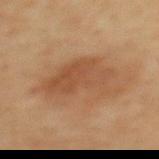Q: Was this lesion biopsied?
A: catalogued during a skin exam; not biopsied
Q: What are the patient's age and sex?
A: female, approximately 55 years of age
Q: Where on the body is the lesion?
A: the back
Q: What is the lesion's diameter?
A: ~8 mm (longest diameter)
Q: How was this image acquired?
A: ~15 mm crop, total-body skin-cancer survey
Q: What lighting was used for the tile?
A: cross-polarized